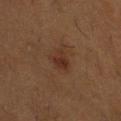Notes:
– workup — catalogued during a skin exam; not biopsied
– lesion diameter — about 3 mm
– TBP lesion metrics — a lesion area of about 4.5 mm², a shape eccentricity near 0.5, and a symmetry-axis asymmetry near 0.35; an average lesion color of about L≈26 a*≈18 b*≈24 (CIELAB), roughly 6 lightness units darker than nearby skin, and a normalized border contrast of about 7; a border-irregularity rating of about 3/10, a within-lesion color-variation index near 3/10, and a peripheral color-asymmetry measure near 1
– patient — male, about 60 years old
– image source — ~15 mm crop, total-body skin-cancer survey
– illumination — cross-polarized